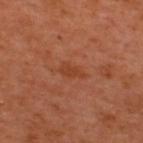biopsy status = catalogued during a skin exam; not biopsied | imaging modality = ~15 mm crop, total-body skin-cancer survey | body site = the upper back | subject = male, aged approximately 50.